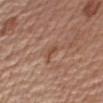Findings:
– notes · total-body-photography surveillance lesion; no biopsy
– imaging modality · ~15 mm crop, total-body skin-cancer survey
– lighting · white-light
– subject · male, approximately 75 years of age
– lesion diameter · ~2.5 mm (longest diameter)
– site · the right upper arm